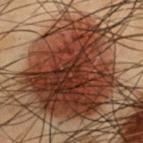biopsy status = total-body-photography surveillance lesion; no biopsy | imaging modality = ~15 mm tile from a whole-body skin photo | lesion size = ≈11 mm | body site = the chest | subject = male, aged around 55 | image-analysis metrics = border irregularity of about 3 on a 0–10 scale and a peripheral color-asymmetry measure near 3 | tile lighting = cross-polarized illumination.